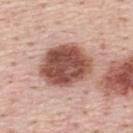{"patient": {"sex": "male", "age_approx": 45}, "image": {"source": "total-body photography crop", "field_of_view_mm": 15}, "site": "back"}